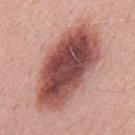Findings:
– biopsy status: total-body-photography surveillance lesion; no biopsy
– subject: male, in their mid- to late 60s
– lesion diameter: about 11.5 mm
– body site: the mid back
– image-analysis metrics: a footprint of about 47 mm² and a symmetry-axis asymmetry near 0.1; a border-irregularity rating of about 2/10, internal color variation of about 9 on a 0–10 scale, and radial color variation of about 2.5; a classifier nevus-likeness of about 30/100 and lesion-presence confidence of about 100/100
– image: ~15 mm crop, total-body skin-cancer survey
– illumination: white-light illumination